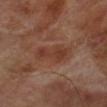• biopsy status · total-body-photography surveillance lesion; no biopsy
• subject · male, about 70 years old
• site · the right lower leg
• acquisition · ~15 mm tile from a whole-body skin photo
• automated metrics · an average lesion color of about L≈37 a*≈22 b*≈27 (CIELAB) and roughly 7 lightness units darker than nearby skin; a within-lesion color-variation index near 3.5/10 and radial color variation of about 1; a nevus-likeness score of about 0/100 and a detector confidence of about 100 out of 100 that the crop contains a lesion